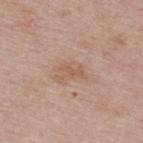{
  "biopsy_status": "not biopsied; imaged during a skin examination"
}